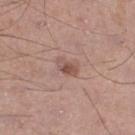Acquisition and patient details: Located on the right lower leg. Imaged with white-light lighting. The total-body-photography lesion software estimated a nevus-likeness score of about 70/100. Longest diameter approximately 3 mm. A 15 mm close-up extracted from a 3D total-body photography capture. The subject is a male in their mid- to late 50s.